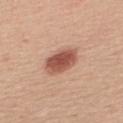patient:
  sex: female
  age_approx: 40
lighting: white-light
automated_metrics:
  eccentricity: 0.75
  shape_asymmetry: 0.15
  cielab_L: 53
  cielab_a: 25
  cielab_b: 29
  vs_skin_darker_L: 15.0
  vs_skin_contrast_norm: 10.0
site: upper back
image:
  source: total-body photography crop
  field_of_view_mm: 15